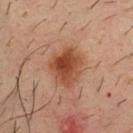Case summary:
- biopsy status · no biopsy performed (imaged during a skin exam)
- lighting · cross-polarized
- image source · ~15 mm crop, total-body skin-cancer survey
- subject · male, aged around 35
- site · the chest
- size · about 5 mm